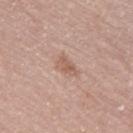biopsy status = total-body-photography surveillance lesion; no biopsy
anatomic site = the left thigh
patient = female, aged around 70
automated metrics = a footprint of about 3.5 mm² and an eccentricity of roughly 0.85
image source = total-body-photography crop, ~15 mm field of view
diameter = ≈3 mm
tile lighting = white-light